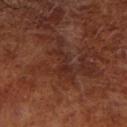Clinical summary: A close-up tile cropped from a whole-body skin photograph, about 15 mm across. A male patient, aged 68–72. On the right lower leg. Automated tile analysis of the lesion measured a classifier nevus-likeness of about 0/100 and a detector confidence of about 80 out of 100 that the crop contains a lesion. The tile uses cross-polarized illumination.